Located on the head or neck, cropped from a total-body skin-imaging series; the visible field is about 15 mm, the subject is a male aged 58 to 62 — 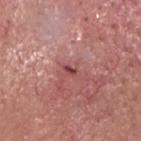Histopathological examination showed a nodular basal cell carcinoma, classified as a malignant lesion.